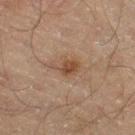Findings:
- follow-up: total-body-photography surveillance lesion; no biopsy
- site: the right thigh
- image source: ~15 mm tile from a whole-body skin photo
- lighting: cross-polarized illumination
- lesion diameter: ≈3 mm
- patient: male, aged 58 to 62